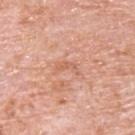biopsy_status: not biopsied; imaged during a skin examination
image:
  source: total-body photography crop
  field_of_view_mm: 15
patient:
  sex: male
  age_approx: 80
site: upper back